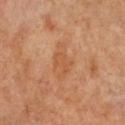Findings:
– acquisition · ~15 mm tile from a whole-body skin photo
– lesion diameter · ~2.5 mm (longest diameter)
– anatomic site · the chest
– patient · female, in their mid-60s
– automated lesion analysis · a shape eccentricity near 0.75 and a symmetry-axis asymmetry near 0.4; a normalized lesion–skin contrast near 5; a detector confidence of about 100 out of 100 that the crop contains a lesion
– tile lighting · cross-polarized